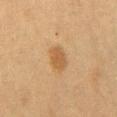Background:
Longest diameter approximately 3 mm. From the abdomen. A 15 mm close-up tile from a total-body photography series done for melanoma screening. A female patient, approximately 40 years of age. The tile uses cross-polarized illumination. Automated tile analysis of the lesion measured an average lesion color of about L≈49 a*≈17 b*≈36 (CIELAB), about 8 CIELAB-L* units darker than the surrounding skin, and a normalized border contrast of about 6.5.